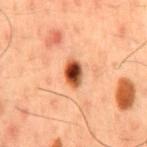Assessment: Captured during whole-body skin photography for melanoma surveillance; the lesion was not biopsied. Acquisition and patient details: The lesion-visualizer software estimated an area of roughly 5.5 mm² and an outline eccentricity of about 0.8 (0 = round, 1 = elongated). The software also gave a mean CIELAB color near L≈38 a*≈24 b*≈31, a lesion–skin lightness drop of about 18, and a lesion-to-skin contrast of about 13.5 (normalized; higher = more distinct). And it measured a border-irregularity index near 1.5/10 and radial color variation of about 2.5. It also reported a classifier nevus-likeness of about 100/100. The patient is a male about 50 years old. A close-up tile cropped from a whole-body skin photograph, about 15 mm across. Approximately 3.5 mm at its widest. Located on the chest. This is a cross-polarized tile.